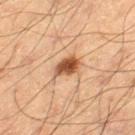Clinical summary:
The patient is a male approximately 65 years of age. The tile uses cross-polarized illumination. Measured at roughly 3.5 mm in maximum diameter. The lesion is on the left thigh. A roughly 15 mm field-of-view crop from a total-body skin photograph.